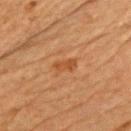Assessment: The lesion was photographed on a routine skin check and not biopsied; there is no pathology result. Clinical summary: Cropped from a total-body skin-imaging series; the visible field is about 15 mm. The recorded lesion diameter is about 2.5 mm. This is a cross-polarized tile. A male patient, aged approximately 65. Located on the front of the torso.